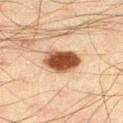Recorded during total-body skin imaging; not selected for excision or biopsy. Automated tile analysis of the lesion measured a mean CIELAB color near L≈43 a*≈20 b*≈30, roughly 19 lightness units darker than nearby skin, and a lesion-to-skin contrast of about 13.5 (normalized; higher = more distinct). A region of skin cropped from a whole-body photographic capture, roughly 15 mm wide. The lesion is on the left thigh. A male patient about 45 years old. The tile uses cross-polarized illumination.